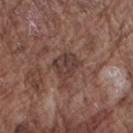lesion size = ≈4 mm
TBP lesion metrics = a lesion area of about 7.5 mm² and two-axis asymmetry of about 0.45; a mean CIELAB color near L≈38 a*≈18 b*≈21; a nevus-likeness score of about 0/100 and a lesion-detection confidence of about 90/100
image = 15 mm crop, total-body photography
subject = male, roughly 75 years of age
lighting = white-light
location = the right upper arm